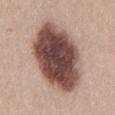biopsy_status: not biopsied; imaged during a skin examination
patient:
  sex: female
  age_approx: 20
image:
  source: total-body photography crop
  field_of_view_mm: 15
site: lower back